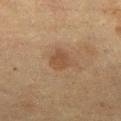biopsy status: total-body-photography surveillance lesion; no biopsy | patient: female, approximately 55 years of age | image: total-body-photography crop, ~15 mm field of view | lighting: cross-polarized | body site: the left thigh | automated metrics: a lesion area of about 5 mm², a shape eccentricity near 0.35, and a symmetry-axis asymmetry near 0.2; a lesion color around L≈39 a*≈15 b*≈27 in CIELAB, about 7 CIELAB-L* units darker than the surrounding skin, and a normalized lesion–skin contrast near 6.